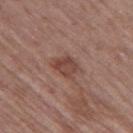Clinical impression: Imaged during a routine full-body skin examination; the lesion was not biopsied and no histopathology is available. Acquisition and patient details: A roughly 15 mm field-of-view crop from a total-body skin photograph. The total-body-photography lesion software estimated a border-irregularity index near 2.5/10, a within-lesion color-variation index near 3.5/10, and peripheral color asymmetry of about 1. It also reported a classifier nevus-likeness of about 30/100. The patient is a male aged 63–67. Located on the left thigh. Approximately 3 mm at its widest.